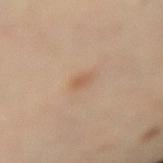Assessment: The lesion was photographed on a routine skin check and not biopsied; there is no pathology result. Image and clinical context: About 1.5 mm across. The subject is a male roughly 55 years of age. The lesion is on the arm. A 15 mm crop from a total-body photograph taken for skin-cancer surveillance. Automated tile analysis of the lesion measured a footprint of about 1.5 mm², a shape eccentricity near 0.65, and a shape-asymmetry score of about 0.2 (0 = symmetric). The analysis additionally found an average lesion color of about L≈57 a*≈19 b*≈33 (CIELAB) and a lesion–skin lightness drop of about 7. The software also gave a border-irregularity rating of about 1.5/10 and a color-variation rating of about 0/10.